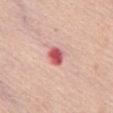Clinical impression: Part of a total-body skin-imaging series; this lesion was reviewed on a skin check and was not flagged for biopsy. Context: On the chest. Measured at roughly 2.5 mm in maximum diameter. A lesion tile, about 15 mm wide, cut from a 3D total-body photograph. An algorithmic analysis of the crop reported a classifier nevus-likeness of about 0/100 and a lesion-detection confidence of about 100/100. The subject is a female aged approximately 75. This is a white-light tile.